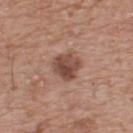Clinical impression: Captured during whole-body skin photography for melanoma surveillance; the lesion was not biopsied. Acquisition and patient details: The total-body-photography lesion software estimated a lesion area of about 8.5 mm², an outline eccentricity of about 0.55 (0 = round, 1 = elongated), and a shape-asymmetry score of about 0.2 (0 = symmetric). The software also gave a normalized lesion–skin contrast near 9.5. The software also gave an automated nevus-likeness rating near 45 out of 100. A male subject, about 75 years old. This is a white-light tile. This image is a 15 mm lesion crop taken from a total-body photograph. The lesion is located on the upper back. The recorded lesion diameter is about 3.5 mm.